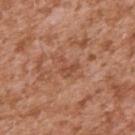Context:
Located on the right upper arm. The tile uses white-light illumination. A close-up tile cropped from a whole-body skin photograph, about 15 mm across. The subject is a male about 45 years old. Measured at roughly 2.5 mm in maximum diameter.